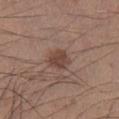The lesion was photographed on a routine skin check and not biopsied; there is no pathology result.
Located on the left lower leg.
A roughly 15 mm field-of-view crop from a total-body skin photograph.
Automated image analysis of the tile measured a shape eccentricity near 0.6 and a symmetry-axis asymmetry near 0.25. The software also gave a border-irregularity rating of about 2/10 and a color-variation rating of about 2/10. And it measured a classifier nevus-likeness of about 80/100 and a detector confidence of about 100 out of 100 that the crop contains a lesion.
The recorded lesion diameter is about 3 mm.
A male subject, in their mid- to late 30s.